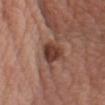The lesion was photographed on a routine skin check and not biopsied; there is no pathology result. The lesion's longest dimension is about 4 mm. On the left upper arm. Cropped from a whole-body photographic skin survey; the tile spans about 15 mm. A male subject, about 55 years old. Imaged with white-light lighting. An algorithmic analysis of the crop reported roughly 15 lightness units darker than nearby skin and a lesion-to-skin contrast of about 11.5 (normalized; higher = more distinct). The software also gave a border-irregularity rating of about 3/10 and internal color variation of about 3.5 on a 0–10 scale. The software also gave an automated nevus-likeness rating near 85 out of 100 and a detector confidence of about 100 out of 100 that the crop contains a lesion.